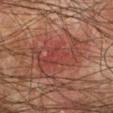Clinical impression:
Part of a total-body skin-imaging series; this lesion was reviewed on a skin check and was not flagged for biopsy.
Image and clinical context:
This is a cross-polarized tile. Automated image analysis of the tile measured a lesion area of about 21 mm² and an outline eccentricity of about 0.8 (0 = round, 1 = elongated). The software also gave an automated nevus-likeness rating near 0 out of 100 and lesion-presence confidence of about 90/100. This image is a 15 mm lesion crop taken from a total-body photograph. A male patient in their mid- to late 70s. The lesion is located on the arm. Measured at roughly 6.5 mm in maximum diameter.